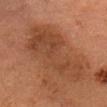Clinical impression: No biopsy was performed on this lesion — it was imaged during a full skin examination and was not determined to be concerning. Context: The lesion is on the head or neck. This is a cross-polarized tile. Automated image analysis of the tile measured a lesion area of about 36 mm². The analysis additionally found a lesion–skin lightness drop of about 8 and a normalized lesion–skin contrast near 6.5. A region of skin cropped from a whole-body photographic capture, roughly 15 mm wide. A female patient, aged approximately 70.